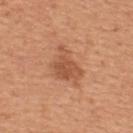image:
  source: total-body photography crop
  field_of_view_mm: 15
automated_metrics:
  cielab_L: 54
  cielab_a: 25
  cielab_b: 35
  vs_skin_darker_L: 10.0
  vs_skin_contrast_norm: 6.5
site: upper back
lighting: white-light
lesion_size:
  long_diameter_mm_approx: 4.5
patient:
  sex: male
  age_approx: 55